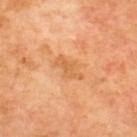Impression: Part of a total-body skin-imaging series; this lesion was reviewed on a skin check and was not flagged for biopsy. Context: This image is a 15 mm lesion crop taken from a total-body photograph. The lesion is located on the upper back. The subject is approximately 65 years of age. The lesion's longest dimension is about 3.5 mm. This is a cross-polarized tile.